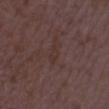This lesion was catalogued during total-body skin photography and was not selected for biopsy.
Automated tile analysis of the lesion measured a footprint of about 3 mm², an eccentricity of roughly 0.9, and two-axis asymmetry of about 0.35. It also reported a border-irregularity index near 3.5/10, a color-variation rating of about 1/10, and a peripheral color-asymmetry measure near 0.
The patient is a female roughly 35 years of age.
A region of skin cropped from a whole-body photographic capture, roughly 15 mm wide.
The lesion is on the leg.